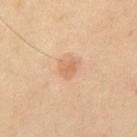Clinical impression: Recorded during total-body skin imaging; not selected for excision or biopsy. Background: Captured under cross-polarized illumination. This image is a 15 mm lesion crop taken from a total-body photograph. A male patient, in their mid-50s. Longest diameter approximately 2.5 mm. Located on the abdomen.